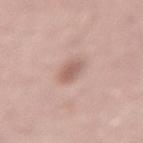biopsy status = imaged on a skin check; not biopsied
subject = male, aged 68–72
anatomic site = the lower back
acquisition = ~15 mm crop, total-body skin-cancer survey